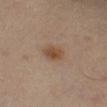Clinical impression:
Captured during whole-body skin photography for melanoma surveillance; the lesion was not biopsied.
Acquisition and patient details:
About 3 mm across. The subject is a male about 65 years old. On the right lower leg. A roughly 15 mm field-of-view crop from a total-body skin photograph.